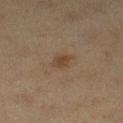Q: Was a biopsy performed?
A: no biopsy performed (imaged during a skin exam)
Q: Patient demographics?
A: female, approximately 40 years of age
Q: What kind of image is this?
A: 15 mm crop, total-body photography
Q: Where on the body is the lesion?
A: the left lower leg